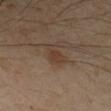No biopsy was performed on this lesion — it was imaged during a full skin examination and was not determined to be concerning. On the left forearm. A 15 mm crop from a total-body photograph taken for skin-cancer surveillance. A female patient, approximately 45 years of age. This is a cross-polarized tile.